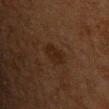Image and clinical context: The lesion's longest dimension is about 3 mm. An algorithmic analysis of the crop reported a lesion area of about 6 mm², a shape eccentricity near 0.65, and a symmetry-axis asymmetry near 0.2. It also reported a mean CIELAB color near L≈19 a*≈14 b*≈21, about 5 CIELAB-L* units darker than the surrounding skin, and a lesion-to-skin contrast of about 6.5 (normalized; higher = more distinct). The tile uses cross-polarized illumination. The subject is a male roughly 65 years of age. A close-up tile cropped from a whole-body skin photograph, about 15 mm across. From the front of the torso.